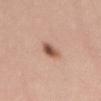Case summary:
– biopsy status · no biopsy performed (imaged during a skin exam)
– imaging modality · ~15 mm tile from a whole-body skin photo
– lighting · white-light illumination
– subject · female, in their mid- to late 20s
– lesion size · ≈3 mm
– location · the abdomen